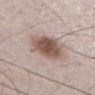A lesion tile, about 15 mm wide, cut from a 3D total-body photograph.
Approximately 5 mm at its widest.
The subject is a male aged 48 to 52.
Located on the abdomen.
Automated image analysis of the tile measured a lesion color around L≈55 a*≈16 b*≈23 in CIELAB, a lesion–skin lightness drop of about 14, and a normalized border contrast of about 9.5. The software also gave a border-irregularity rating of about 2/10 and internal color variation of about 6 on a 0–10 scale. And it measured a nevus-likeness score of about 100/100 and lesion-presence confidence of about 100/100.